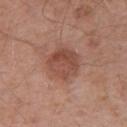follow-up = total-body-photography surveillance lesion; no biopsy | image = total-body-photography crop, ~15 mm field of view | illumination = white-light illumination | subject = male, roughly 55 years of age | lesion diameter = about 4 mm | automated metrics = a footprint of about 11 mm², an eccentricity of roughly 0.25, and two-axis asymmetry of about 0.15; about 9 CIELAB-L* units darker than the surrounding skin and a normalized border contrast of about 7; a classifier nevus-likeness of about 35/100 | body site = the right upper arm.